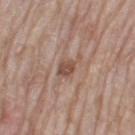The lesion was tiled from a total-body skin photograph and was not biopsied.